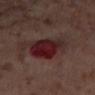Clinical impression: Imaged during a routine full-body skin examination; the lesion was not biopsied and no histopathology is available. Background: A female subject roughly 55 years of age. The lesion's longest dimension is about 5 mm. The tile uses cross-polarized illumination. The lesion-visualizer software estimated a footprint of about 16 mm², an outline eccentricity of about 0.5 (0 = round, 1 = elongated), and two-axis asymmetry of about 0.2. The analysis additionally found an average lesion color of about L≈23 a*≈24 b*≈17 (CIELAB), roughly 10 lightness units darker than nearby skin, and a lesion-to-skin contrast of about 11 (normalized; higher = more distinct). The software also gave peripheral color asymmetry of about 3. On the left thigh. Cropped from a whole-body photographic skin survey; the tile spans about 15 mm.